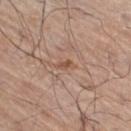Impression: Recorded during total-body skin imaging; not selected for excision or biopsy. Image and clinical context: A 15 mm crop from a total-body photograph taken for skin-cancer surveillance. A male subject, aged approximately 70. From the right thigh. Longest diameter approximately 2.5 mm. Automated image analysis of the tile measured an average lesion color of about L≈53 a*≈19 b*≈30 (CIELAB), about 8 CIELAB-L* units darker than the surrounding skin, and a normalized border contrast of about 6.5. This is a white-light tile.